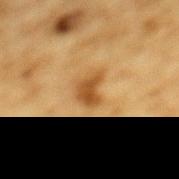Captured during whole-body skin photography for melanoma surveillance; the lesion was not biopsied. On the mid back. A male patient approximately 85 years of age or older. Approximately 3 mm at its widest. A region of skin cropped from a whole-body photographic capture, roughly 15 mm wide. The tile uses cross-polarized illumination.